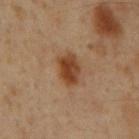workup = no biopsy performed (imaged during a skin exam).